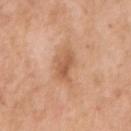Case summary:
- biopsy status — no biopsy performed (imaged during a skin exam)
- lighting — white-light
- anatomic site — the right upper arm
- image-analysis metrics — a lesion-to-skin contrast of about 6.5 (normalized; higher = more distinct); a detector confidence of about 100 out of 100 that the crop contains a lesion
- lesion size — ≈3.5 mm
- patient — female, roughly 55 years of age
- imaging modality — 15 mm crop, total-body photography